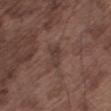biopsy status: catalogued during a skin exam; not biopsied
patient: male, in their mid- to late 70s
lesion size: ~2.5 mm (longest diameter)
site: the leg
lighting: white-light
TBP lesion metrics: an area of roughly 3.5 mm², an outline eccentricity of about 0.85 (0 = round, 1 = elongated), and a shape-asymmetry score of about 0.4 (0 = symmetric); a lesion color around L≈37 a*≈16 b*≈21 in CIELAB and a normalized lesion–skin contrast near 6
imaging modality: 15 mm crop, total-body photography